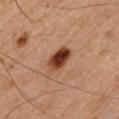Case summary:
* lesion size — ~4 mm (longest diameter)
* subject — male, aged 43–47
* anatomic site — the left upper arm
* image source — ~15 mm crop, total-body skin-cancer survey
* tile lighting — cross-polarized